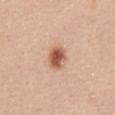Notes:
– follow-up — total-body-photography surveillance lesion; no biopsy
– automated lesion analysis — roughly 15 lightness units darker than nearby skin; a border-irregularity index near 1.5/10, a color-variation rating of about 4.5/10, and radial color variation of about 1; an automated nevus-likeness rating near 100 out of 100 and lesion-presence confidence of about 100/100
– subject — female, in their mid-40s
– imaging modality — ~15 mm crop, total-body skin-cancer survey
– diameter — about 3 mm
– illumination — white-light illumination
– location — the front of the torso